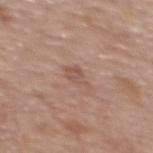Clinical impression: No biopsy was performed on this lesion — it was imaged during a full skin examination and was not determined to be concerning. Acquisition and patient details: The recorded lesion diameter is about 3 mm. On the upper back. This image is a 15 mm lesion crop taken from a total-body photograph. The lesion-visualizer software estimated a within-lesion color-variation index near 2/10 and a peripheral color-asymmetry measure near 0.5. The software also gave a nevus-likeness score of about 0/100 and a lesion-detection confidence of about 100/100. A female patient aged 53 to 57.